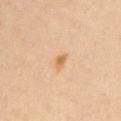Case summary:
- notes: no biopsy performed (imaged during a skin exam)
- imaging modality: ~15 mm tile from a whole-body skin photo
- illumination: cross-polarized
- location: the left upper arm
- lesion size: ~2 mm (longest diameter)
- automated lesion analysis: an area of roughly 2 mm², an eccentricity of roughly 0.65, and two-axis asymmetry of about 0.2; border irregularity of about 2 on a 0–10 scale and peripheral color asymmetry of about 0.5; lesion-presence confidence of about 100/100
- subject: female, aged 28–32